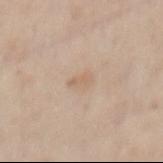{"biopsy_status": "not biopsied; imaged during a skin examination", "site": "mid back", "automated_metrics": {"area_mm2_approx": 3.5, "eccentricity": 0.8, "shape_asymmetry": 0.25, "border_irregularity_0_10": 2.5, "color_variation_0_10": 1.5, "peripheral_color_asymmetry": 0.5, "nevus_likeness_0_100": 0, "lesion_detection_confidence_0_100": 100}, "patient": {"sex": "female", "age_approx": 40}, "image": {"source": "total-body photography crop", "field_of_view_mm": 15}}